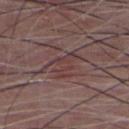* follow-up — catalogued during a skin exam; not biopsied
* image source — 15 mm crop, total-body photography
* illumination — white-light
* patient — male, aged around 50
* size — ≈4.5 mm
* anatomic site — the chest